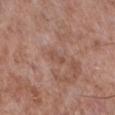The lesion was photographed on a routine skin check and not biopsied; there is no pathology result.
The patient is a male aged around 55.
Cropped from a total-body skin-imaging series; the visible field is about 15 mm.
Captured under white-light illumination.
On the right lower leg.